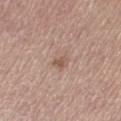Imaged during a routine full-body skin examination; the lesion was not biopsied and no histopathology is available. Imaged with white-light lighting. This image is a 15 mm lesion crop taken from a total-body photograph. The subject is a female about 65 years old. Approximately 3 mm at its widest. On the left thigh.